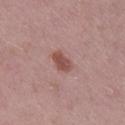Case summary:
* workup · imaged on a skin check; not biopsied
* body site · the right lower leg
* image · total-body-photography crop, ~15 mm field of view
* subject · male, aged approximately 40
* size · ≈3 mm
* illumination · white-light illumination